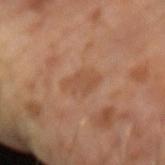* imaging modality: 15 mm crop, total-body photography
* tile lighting: cross-polarized illumination
* patient: male, aged 63 to 67
* lesion diameter: about 4 mm